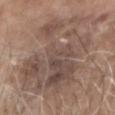The lesion was photographed on a routine skin check and not biopsied; there is no pathology result.
A 15 mm crop from a total-body photograph taken for skin-cancer surveillance.
Approximately 11.5 mm at its widest.
Automated tile analysis of the lesion measured a footprint of about 70 mm², an outline eccentricity of about 0.65 (0 = round, 1 = elongated), and two-axis asymmetry of about 0.35. It also reported a mean CIELAB color near L≈49 a*≈15 b*≈23 and a lesion-to-skin contrast of about 7 (normalized; higher = more distinct). And it measured a border-irregularity rating of about 7.5/10, a color-variation rating of about 7/10, and radial color variation of about 2.5.
A male subject, in their 80s.
On the head or neck.
Imaged with white-light lighting.